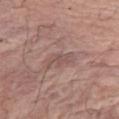notes = imaged on a skin check; not biopsied
lighting = white-light illumination
imaging modality = 15 mm crop, total-body photography
body site = the chest
subject = female, in their mid- to late 60s
lesion diameter = ~3.5 mm (longest diameter)